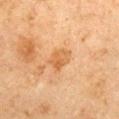Captured during whole-body skin photography for melanoma surveillance; the lesion was not biopsied.
From the arm.
A 15 mm close-up tile from a total-body photography series done for melanoma screening.
Imaged with cross-polarized lighting.
About 3 mm across.
The patient is a male aged around 65.
The lesion-visualizer software estimated a normalized lesion–skin contrast near 6.5. It also reported a nevus-likeness score of about 0/100 and lesion-presence confidence of about 100/100.